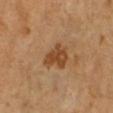This lesion was catalogued during total-body skin photography and was not selected for biopsy. The recorded lesion diameter is about 4 mm. Cropped from a whole-body photographic skin survey; the tile spans about 15 mm. A female patient, in their mid-50s. Located on the right lower leg.